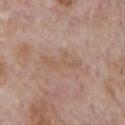Captured during whole-body skin photography for melanoma surveillance; the lesion was not biopsied. A 15 mm close-up extracted from a 3D total-body photography capture. Imaged with white-light lighting. The subject is a male aged approximately 70. Approximately 5 mm at its widest. The lesion is on the chest.